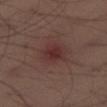  biopsy_status: not biopsied; imaged during a skin examination
  image:
    source: total-body photography crop
    field_of_view_mm: 15
  patient:
    sex: male
    age_approx: 40
  site: left thigh